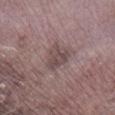| key | value |
|---|---|
| workup | catalogued during a skin exam; not biopsied |
| image-analysis metrics | an average lesion color of about L≈46 a*≈15 b*≈16 (CIELAB), roughly 8 lightness units darker than nearby skin, and a lesion-to-skin contrast of about 6.5 (normalized; higher = more distinct); a classifier nevus-likeness of about 0/100 |
| image source | total-body-photography crop, ~15 mm field of view |
| anatomic site | the right lower leg |
| subject | male, in their mid-70s |